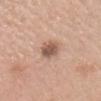Clinical impression:
Recorded during total-body skin imaging; not selected for excision or biopsy.
Acquisition and patient details:
The subject is a female roughly 40 years of age. A region of skin cropped from a whole-body photographic capture, roughly 15 mm wide. The lesion is located on the head or neck. Approximately 2.5 mm at its widest. The tile uses white-light illumination.